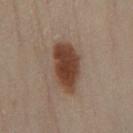biopsy status=imaged on a skin check; not biopsied | site=the left leg | image source=total-body-photography crop, ~15 mm field of view | image-analysis metrics=a lesion color around L≈42 a*≈19 b*≈27 in CIELAB and a normalized lesion–skin contrast near 11.5 | lighting=cross-polarized | subject=female, aged approximately 40.